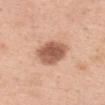The lesion was photographed on a routine skin check and not biopsied; there is no pathology result. The patient is a female aged 48 to 52. From the back. This image is a 15 mm lesion crop taken from a total-body photograph.